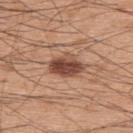Findings:
• notes — no biopsy performed (imaged during a skin exam)
• patient — male, about 60 years old
• imaging modality — 15 mm crop, total-body photography
• site — the upper back
• tile lighting — white-light illumination
• image-analysis metrics — an eccentricity of roughly 0.8 and two-axis asymmetry of about 0.2; an average lesion color of about L≈47 a*≈22 b*≈29 (CIELAB), about 15 CIELAB-L* units darker than the surrounding skin, and a normalized lesion–skin contrast near 10.5